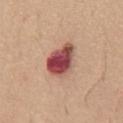Assessment:
Captured during whole-body skin photography for melanoma surveillance; the lesion was not biopsied.
Image and clinical context:
The lesion is on the chest. A male subject approximately 60 years of age. Measured at roughly 4.5 mm in maximum diameter. Captured under white-light illumination. A close-up tile cropped from a whole-body skin photograph, about 15 mm across.